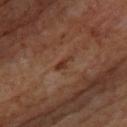Background:
From the upper back. The subject is a male about 70 years old. This is a cross-polarized tile. A 15 mm crop from a total-body photograph taken for skin-cancer surveillance.